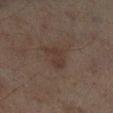Assessment:
Captured during whole-body skin photography for melanoma surveillance; the lesion was not biopsied.
Acquisition and patient details:
The lesion's longest dimension is about 3.5 mm. Located on the left lower leg. A male subject about 70 years old. A roughly 15 mm field-of-view crop from a total-body skin photograph. Captured under cross-polarized illumination.